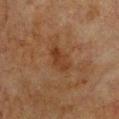follow-up = no biopsy performed (imaged during a skin exam)
lesion size = ~3.5 mm (longest diameter)
lighting = cross-polarized illumination
imaging modality = total-body-photography crop, ~15 mm field of view
patient = female, about 55 years old
anatomic site = the chest